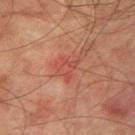Impression:
No biopsy was performed on this lesion — it was imaged during a full skin examination and was not determined to be concerning.
Acquisition and patient details:
From the left upper arm. A close-up tile cropped from a whole-body skin photograph, about 15 mm across. The recorded lesion diameter is about 3.5 mm. A male patient aged around 75. Captured under cross-polarized illumination.